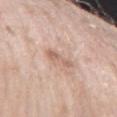Captured during whole-body skin photography for melanoma surveillance; the lesion was not biopsied. Captured under white-light illumination. This image is a 15 mm lesion crop taken from a total-body photograph. Approximately 3 mm at its widest. From the left forearm. The subject is a female approximately 70 years of age.